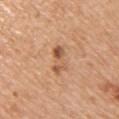notes: catalogued during a skin exam; not biopsied
TBP lesion metrics: a peripheral color-asymmetry measure near 3.5; a nevus-likeness score of about 45/100 and a detector confidence of about 100 out of 100 that the crop contains a lesion
illumination: white-light illumination
patient: female, aged 53–57
acquisition: ~15 mm tile from a whole-body skin photo
location: the right upper arm